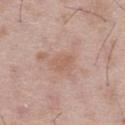biopsy status: no biopsy performed (imaged during a skin exam) | imaging modality: ~15 mm crop, total-body skin-cancer survey | image-analysis metrics: a footprint of about 4 mm², a shape eccentricity near 0.7, and a shape-asymmetry score of about 0.3 (0 = symmetric); border irregularity of about 3 on a 0–10 scale, a color-variation rating of about 1.5/10, and a peripheral color-asymmetry measure near 0.5 | location: the right thigh | subject: male, about 50 years old | lesion diameter: about 2.5 mm.